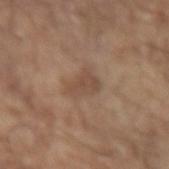Clinical impression:
The lesion was photographed on a routine skin check and not biopsied; there is no pathology result.